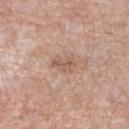{
  "biopsy_status": "not biopsied; imaged during a skin examination",
  "patient": {
    "sex": "female",
    "age_approx": 70
  },
  "site": "left lower leg",
  "image": {
    "source": "total-body photography crop",
    "field_of_view_mm": 15
  }
}